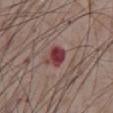follow-up: no biopsy performed (imaged during a skin exam); lesion diameter: about 2.5 mm; anatomic site: the abdomen; acquisition: total-body-photography crop, ~15 mm field of view; subject: male, aged 73–77; lighting: white-light.